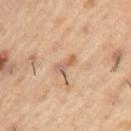| key | value |
|---|---|
| follow-up | total-body-photography surveillance lesion; no biopsy |
| patient | male, aged approximately 55 |
| location | the arm |
| lesion size | ~3 mm (longest diameter) |
| imaging modality | ~15 mm tile from a whole-body skin photo |
| automated metrics | an eccentricity of roughly 0.9 and a shape-asymmetry score of about 0.35 (0 = symmetric) |